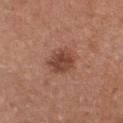Assessment: Part of a total-body skin-imaging series; this lesion was reviewed on a skin check and was not flagged for biopsy. Acquisition and patient details: A 15 mm crop from a total-body photograph taken for skin-cancer surveillance. Longest diameter approximately 3.5 mm. The patient is a female aged approximately 30. Located on the chest.